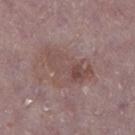{
  "biopsy_status": "not biopsied; imaged during a skin examination",
  "lighting": "white-light",
  "image": {
    "source": "total-body photography crop",
    "field_of_view_mm": 15
  },
  "automated_metrics": {
    "area_mm2_approx": 17.0,
    "shape_asymmetry": 0.45
  },
  "lesion_size": {
    "long_diameter_mm_approx": 6.5
  },
  "patient": {
    "sex": "male",
    "age_approx": 75
  },
  "site": "right lower leg"
}